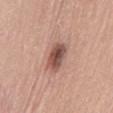Q: Was this lesion biopsied?
A: catalogued during a skin exam; not biopsied
Q: What kind of image is this?
A: 15 mm crop, total-body photography
Q: What did automated image analysis measure?
A: a footprint of about 8 mm², an eccentricity of roughly 0.8, and two-axis asymmetry of about 0.2; a mean CIELAB color near L≈51 a*≈22 b*≈25, about 15 CIELAB-L* units darker than the surrounding skin, and a normalized lesion–skin contrast near 10; border irregularity of about 2 on a 0–10 scale and peripheral color asymmetry of about 2
Q: Lesion location?
A: the lower back
Q: Patient demographics?
A: male, in their mid-40s
Q: How was the tile lit?
A: white-light
Q: Lesion size?
A: ~4 mm (longest diameter)